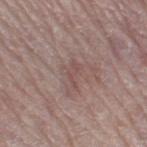{
  "biopsy_status": "not biopsied; imaged during a skin examination",
  "lesion_size": {
    "long_diameter_mm_approx": 3.0
  },
  "patient": {
    "sex": "male",
    "age_approx": 80
  },
  "site": "right thigh",
  "image": {
    "source": "total-body photography crop",
    "field_of_view_mm": 15
  }
}